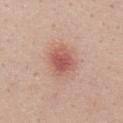Q: Was a biopsy performed?
A: total-body-photography surveillance lesion; no biopsy